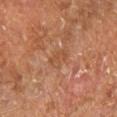The lesion was photographed on a routine skin check and not biopsied; there is no pathology result. A 15 mm close-up extracted from a 3D total-body photography capture. A male subject aged approximately 60. Captured under cross-polarized illumination. On the right leg. Longest diameter approximately 3 mm.